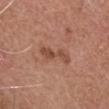Clinical impression:
No biopsy was performed on this lesion — it was imaged during a full skin examination and was not determined to be concerning.
Background:
Captured under white-light illumination. The subject is a male about 75 years old. The lesion is located on the head or neck. Approximately 4.5 mm at its widest. Automated tile analysis of the lesion measured an eccentricity of roughly 0.95 and a shape-asymmetry score of about 0.3 (0 = symmetric). And it measured a lesion color around L≈48 a*≈23 b*≈30 in CIELAB, a lesion–skin lightness drop of about 9, and a normalized border contrast of about 7. The software also gave border irregularity of about 5 on a 0–10 scale and a peripheral color-asymmetry measure near 1. It also reported a classifier nevus-likeness of about 5/100. A 15 mm crop from a total-body photograph taken for skin-cancer surveillance.